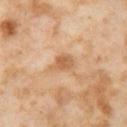patient=female, in their mid- to late 50s
lighting=cross-polarized illumination
lesion size=~3 mm (longest diameter)
anatomic site=the left thigh
image-analysis metrics=a footprint of about 5 mm² and two-axis asymmetry of about 0.35; an average lesion color of about L≈62 a*≈21 b*≈38 (CIELAB)
imaging modality=~15 mm crop, total-body skin-cancer survey